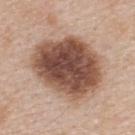Assessment:
The lesion was photographed on a routine skin check and not biopsied; there is no pathology result.
Context:
Located on the back. Measured at roughly 8.5 mm in maximum diameter. A lesion tile, about 15 mm wide, cut from a 3D total-body photograph. This is a white-light tile. The subject is a female aged 43–47. Automated image analysis of the tile measured an area of roughly 45 mm², a shape eccentricity near 0.65, and a shape-asymmetry score of about 0.1 (0 = symmetric).